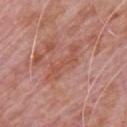- biopsy status: no biopsy performed (imaged during a skin exam)
- automated lesion analysis: a mean CIELAB color near L≈53 a*≈26 b*≈30 and roughly 7 lightness units darker than nearby skin; a border-irregularity rating of about 9/10, a color-variation rating of about 0.5/10, and peripheral color asymmetry of about 0; a nevus-likeness score of about 0/100 and a lesion-detection confidence of about 75/100
- lesion size: about 5.5 mm
- site: the chest
- patient: male, aged approximately 80
- image: ~15 mm tile from a whole-body skin photo
- lighting: white-light illumination